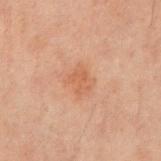Part of a total-body skin-imaging series; this lesion was reviewed on a skin check and was not flagged for biopsy. The lesion is located on the chest. The recorded lesion diameter is about 3 mm. A male patient, aged 58–62. A 15 mm crop from a total-body photograph taken for skin-cancer surveillance. Automated image analysis of the tile measured an area of roughly 6 mm² and two-axis asymmetry of about 0.25. It also reported a border-irregularity index near 2.5/10, a within-lesion color-variation index near 2/10, and peripheral color asymmetry of about 0.5. It also reported a nevus-likeness score of about 0/100.